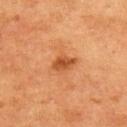Q: What is the imaging modality?
A: ~15 mm tile from a whole-body skin photo
Q: What are the patient's age and sex?
A: male, aged 73 to 77
Q: Automated lesion metrics?
A: a mean CIELAB color near L≈41 a*≈25 b*≈36, a lesion–skin lightness drop of about 10, and a normalized lesion–skin contrast near 8
Q: Where on the body is the lesion?
A: the back